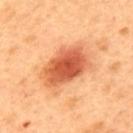<lesion>
  <biopsy_status>not biopsied; imaged during a skin examination</biopsy_status>
  <lighting>cross-polarized</lighting>
  <lesion_size>
    <long_diameter_mm_approx>6.5</long_diameter_mm_approx>
  </lesion_size>
  <image>
    <source>total-body photography crop</source>
    <field_of_view_mm>15</field_of_view_mm>
  </image>
  <patient>
    <sex>male</sex>
    <age_approx>50</age_approx>
  </patient>
  <site>upper back</site>
  <automated_metrics>
    <border_irregularity_0_10>2.0</border_irregularity_0_10>
    <color_variation_0_10>6.0</color_variation_0_10>
    <peripheral_color_asymmetry>1.5</peripheral_color_asymmetry>
  </automated_metrics>
</lesion>